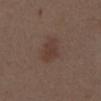Q: Was a biopsy performed?
A: no biopsy performed (imaged during a skin exam)
Q: Where on the body is the lesion?
A: the mid back
Q: What is the lesion's diameter?
A: ≈3.5 mm
Q: Patient demographics?
A: male, aged approximately 70
Q: How was this image acquired?
A: total-body-photography crop, ~15 mm field of view
Q: What lighting was used for the tile?
A: white-light illumination
Q: Automated lesion metrics?
A: a footprint of about 6 mm², an eccentricity of roughly 0.75, and a symmetry-axis asymmetry near 0.3; an average lesion color of about L≈37 a*≈17 b*≈22 (CIELAB), a lesion–skin lightness drop of about 6, and a normalized lesion–skin contrast near 6; border irregularity of about 3 on a 0–10 scale and peripheral color asymmetry of about 0.5; a classifier nevus-likeness of about 80/100 and a lesion-detection confidence of about 100/100